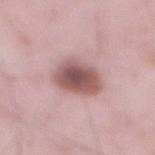Captured during whole-body skin photography for melanoma surveillance; the lesion was not biopsied. The subject is a male aged around 55. A 15 mm close-up extracted from a 3D total-body photography capture. This is a white-light tile. The lesion's longest dimension is about 4.5 mm. The total-body-photography lesion software estimated a lesion–skin lightness drop of about 15 and a normalized border contrast of about 10. It also reported a border-irregularity rating of about 2/10 and a color-variation rating of about 5/10. The lesion is located on the abdomen.